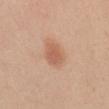Part of a total-body skin-imaging series; this lesion was reviewed on a skin check and was not flagged for biopsy.
The tile uses white-light illumination.
This image is a 15 mm lesion crop taken from a total-body photograph.
A female subject, aged approximately 45.
The lesion-visualizer software estimated an area of roughly 6.5 mm², a shape eccentricity near 0.7, and a shape-asymmetry score of about 0.15 (0 = symmetric). It also reported border irregularity of about 1.5 on a 0–10 scale. The analysis additionally found a classifier nevus-likeness of about 95/100 and a lesion-detection confidence of about 100/100.
Located on the mid back.